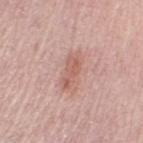workup=imaged on a skin check; not biopsied | image source=~15 mm crop, total-body skin-cancer survey | lesion diameter=~4 mm (longest diameter) | anatomic site=the arm | image-analysis metrics=a lesion color around L≈59 a*≈23 b*≈26 in CIELAB, about 9 CIELAB-L* units darker than the surrounding skin, and a normalized lesion–skin contrast near 6; border irregularity of about 3.5 on a 0–10 scale and a peripheral color-asymmetry measure near 1 | tile lighting=white-light illumination | subject=female, aged 63 to 67.